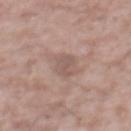Notes:
* notes — total-body-photography surveillance lesion; no biopsy
* size — ~3.5 mm (longest diameter)
* subject — male, aged approximately 45
* body site — the mid back
* tile lighting — white-light
* acquisition — ~15 mm tile from a whole-body skin photo
* image-analysis metrics — a footprint of about 4.5 mm², an eccentricity of roughly 0.85, and two-axis asymmetry of about 0.3; a border-irregularity rating of about 3/10, internal color variation of about 2 on a 0–10 scale, and peripheral color asymmetry of about 0.5; an automated nevus-likeness rating near 0 out of 100 and a lesion-detection confidence of about 100/100